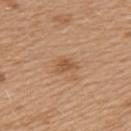Captured during whole-body skin photography for melanoma surveillance; the lesion was not biopsied. A 15 mm close-up tile from a total-body photography series done for melanoma screening. The lesion is on the left upper arm. Measured at roughly 2.5 mm in maximum diameter. The lesion-visualizer software estimated an area of roughly 3.5 mm², an eccentricity of roughly 0.8, and two-axis asymmetry of about 0.3. The software also gave roughly 9 lightness units darker than nearby skin and a normalized lesion–skin contrast near 6.5. The software also gave a nevus-likeness score of about 30/100. A female subject, aged 38 to 42.